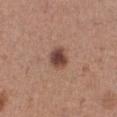Impression:
Part of a total-body skin-imaging series; this lesion was reviewed on a skin check and was not flagged for biopsy.
Image and clinical context:
An algorithmic analysis of the crop reported an average lesion color of about L≈43 a*≈21 b*≈25 (CIELAB), a lesion–skin lightness drop of about 15, and a normalized lesion–skin contrast near 11. The analysis additionally found a border-irregularity rating of about 1.5/10, a within-lesion color-variation index near 5/10, and a peripheral color-asymmetry measure near 2. It also reported a nevus-likeness score of about 95/100 and lesion-presence confidence of about 100/100. A female subject, roughly 30 years of age. The lesion's longest dimension is about 2.5 mm. A roughly 15 mm field-of-view crop from a total-body skin photograph. On the right lower leg. This is a white-light tile.